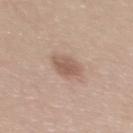Case summary:
• acquisition · ~15 mm tile from a whole-body skin photo
• automated lesion analysis · an automated nevus-likeness rating near 50 out of 100 and lesion-presence confidence of about 100/100
• diameter · ≈3.5 mm
• anatomic site · the upper back
• patient · female, about 35 years old
• lighting · white-light illumination